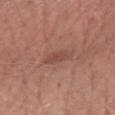Impression:
Recorded during total-body skin imaging; not selected for excision or biopsy.
Acquisition and patient details:
Automated image analysis of the tile measured an eccentricity of roughly 0.75 and a shape-asymmetry score of about 0.25 (0 = symmetric). The analysis additionally found border irregularity of about 2.5 on a 0–10 scale. It also reported a classifier nevus-likeness of about 0/100 and lesion-presence confidence of about 100/100. Measured at roughly 2.5 mm in maximum diameter. A close-up tile cropped from a whole-body skin photograph, about 15 mm across. Captured under white-light illumination. The patient is a female in their 60s. From the right forearm.